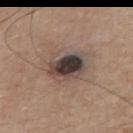imaging modality = 15 mm crop, total-body photography
diameter = ≈5 mm
location = the mid back
lighting = white-light illumination
patient = male, in their mid-70s